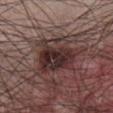biopsy status — catalogued during a skin exam; not biopsied
lesion size — ≈5 mm
body site — the chest
TBP lesion metrics — a border-irregularity rating of about 3/10, a color-variation rating of about 8.5/10, and radial color variation of about 3
image source — ~15 mm crop, total-body skin-cancer survey
patient — male, aged 63 to 67
tile lighting — white-light illumination